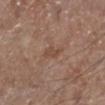The lesion was tiled from a total-body skin photograph and was not biopsied.
A male patient in their mid-60s.
Measured at roughly 2.5 mm in maximum diameter.
From the left lower leg.
A 15 mm close-up extracted from a 3D total-body photography capture.
The lesion-visualizer software estimated a mean CIELAB color near L≈47 a*≈19 b*≈27, roughly 6 lightness units darker than nearby skin, and a normalized lesion–skin contrast near 5.5. The software also gave border irregularity of about 3.5 on a 0–10 scale, internal color variation of about 0 on a 0–10 scale, and radial color variation of about 0. The software also gave a nevus-likeness score of about 0/100 and a detector confidence of about 100 out of 100 that the crop contains a lesion.
The tile uses white-light illumination.